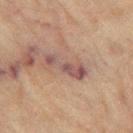notes=total-body-photography surveillance lesion; no biopsy | body site=the left thigh | image source=total-body-photography crop, ~15 mm field of view | subject=female, aged approximately 80 | automated lesion analysis=a lesion area of about 8 mm², an eccentricity of roughly 0.95, and a symmetry-axis asymmetry near 0.35; a border-irregularity index near 5.5/10, a color-variation rating of about 3/10, and a peripheral color-asymmetry measure near 1; an automated nevus-likeness rating near 0 out of 100 and lesion-presence confidence of about 85/100 | illumination=cross-polarized illumination | size=~5 mm (longest diameter).